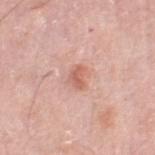Part of a total-body skin-imaging series; this lesion was reviewed on a skin check and was not flagged for biopsy. An algorithmic analysis of the crop reported an automated nevus-likeness rating near 10 out of 100 and lesion-presence confidence of about 100/100. On the right thigh. A 15 mm crop from a total-body photograph taken for skin-cancer surveillance. Approximately 2.5 mm at its widest. A male subject, approximately 80 years of age. The tile uses white-light illumination.